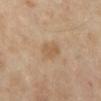Clinical impression: The lesion was tiled from a total-body skin photograph and was not biopsied. Clinical summary: A female subject in their 70s. The lesion is located on the leg. The lesion's longest dimension is about 2.5 mm. Imaged with cross-polarized lighting. The lesion-visualizer software estimated an eccentricity of roughly 0.7. The software also gave a mean CIELAB color near L≈58 a*≈16 b*≈35, about 7 CIELAB-L* units darker than the surrounding skin, and a normalized border contrast of about 5.5. It also reported an automated nevus-likeness rating near 5 out of 100. Cropped from a whole-body photographic skin survey; the tile spans about 15 mm.